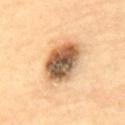The lesion was tiled from a total-body skin photograph and was not biopsied.
The lesion's longest dimension is about 5.5 mm.
A 15 mm crop from a total-body photograph taken for skin-cancer surveillance.
A male subject aged 83 or older.
The total-body-photography lesion software estimated a mean CIELAB color near L≈56 a*≈18 b*≈35, about 21 CIELAB-L* units darker than the surrounding skin, and a normalized border contrast of about 12.5. The analysis additionally found a border-irregularity rating of about 1.5/10, a color-variation rating of about 10/10, and radial color variation of about 4.5. And it measured a classifier nevus-likeness of about 5/100.
Imaged with cross-polarized lighting.
The lesion is on the upper back.